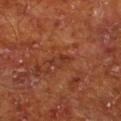This lesion was catalogued during total-body skin photography and was not selected for biopsy. About 2.5 mm across. On the left lower leg. A lesion tile, about 15 mm wide, cut from a 3D total-body photograph. Captured under cross-polarized illumination. Automated image analysis of the tile measured a footprint of about 3 mm² and a shape eccentricity near 0.85. And it measured an average lesion color of about L≈34 a*≈26 b*≈30 (CIELAB), a lesion–skin lightness drop of about 6, and a normalized lesion–skin contrast near 5.5. And it measured a within-lesion color-variation index near 0/10 and a peripheral color-asymmetry measure near 0. The subject is a male aged 63 to 67.